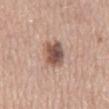Recorded during total-body skin imaging; not selected for excision or biopsy. This is a white-light tile. A male subject roughly 65 years of age. A 15 mm crop from a total-body photograph taken for skin-cancer surveillance. Located on the mid back. The total-body-photography lesion software estimated an eccentricity of roughly 0.75 and a symmetry-axis asymmetry near 0.2. And it measured border irregularity of about 2.5 on a 0–10 scale, a within-lesion color-variation index near 7/10, and peripheral color asymmetry of about 2.